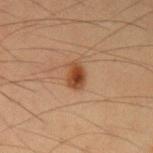The lesion is on the left upper arm.
A male subject, aged approximately 35.
A close-up tile cropped from a whole-body skin photograph, about 15 mm across.